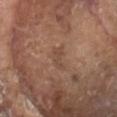The lesion was tiled from a total-body skin photograph and was not biopsied. On the head or neck. Longest diameter approximately 3 mm. The patient is a male aged approximately 80. A lesion tile, about 15 mm wide, cut from a 3D total-body photograph. Captured under white-light illumination.